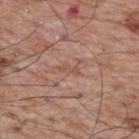Q: Was a biopsy performed?
A: imaged on a skin check; not biopsied
Q: Lesion location?
A: the upper back
Q: What did automated image analysis measure?
A: a shape eccentricity near 0.9 and a shape-asymmetry score of about 0.35 (0 = symmetric); a border-irregularity rating of about 3.5/10, a color-variation rating of about 0/10, and a peripheral color-asymmetry measure near 0; a nevus-likeness score of about 0/100 and lesion-presence confidence of about 60/100
Q: What are the patient's age and sex?
A: male, aged approximately 70
Q: What is the imaging modality?
A: total-body-photography crop, ~15 mm field of view